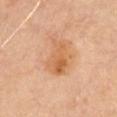The subject is a male approximately 65 years of age.
The tile uses cross-polarized illumination.
Cropped from a total-body skin-imaging series; the visible field is about 15 mm.
An algorithmic analysis of the crop reported a lesion color around L≈58 a*≈22 b*≈38 in CIELAB, a lesion–skin lightness drop of about 8, and a normalized lesion–skin contrast near 6.5. The analysis additionally found border irregularity of about 3 on a 0–10 scale, a color-variation rating of about 6/10, and peripheral color asymmetry of about 2. The analysis additionally found a classifier nevus-likeness of about 20/100 and lesion-presence confidence of about 100/100.
The lesion is located on the front of the torso.
Measured at roughly 4.5 mm in maximum diameter.